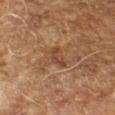biopsy status — no biopsy performed (imaged during a skin exam); patient — male, aged 68–72; image source — ~15 mm tile from a whole-body skin photo; lesion diameter — about 3 mm; anatomic site — the right forearm.